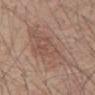Notes:
– workup — catalogued during a skin exam; not biopsied
– image — total-body-photography crop, ~15 mm field of view
– subject — male, aged 78 to 82
– illumination — white-light
– lesion size — ~7 mm (longest diameter)
– location — the abdomen
– automated lesion analysis — an area of roughly 29 mm², an outline eccentricity of about 0.6 (0 = round, 1 = elongated), and a shape-asymmetry score of about 0.25 (0 = symmetric)